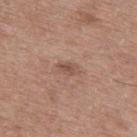* follow-up — imaged on a skin check; not biopsied
* lesion size — ~2.5 mm (longest diameter)
* patient — male, about 60 years old
* illumination — white-light illumination
* body site — the upper back
* imaging modality — ~15 mm tile from a whole-body skin photo